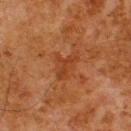{
  "biopsy_status": "not biopsied; imaged during a skin examination",
  "automated_metrics": {
    "border_irregularity_0_10": 5.0,
    "color_variation_0_10": 2.5,
    "peripheral_color_asymmetry": 1.0,
    "nevus_likeness_0_100": 0,
    "lesion_detection_confidence_0_100": 100
  },
  "lighting": "cross-polarized",
  "site": "upper back",
  "lesion_size": {
    "long_diameter_mm_approx": 3.5
  },
  "image": {
    "source": "total-body photography crop",
    "field_of_view_mm": 15
  },
  "patient": {
    "sex": "male",
    "age_approx": 80
  }
}